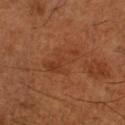Recorded during total-body skin imaging; not selected for excision or biopsy. Automated image analysis of the tile measured a nevus-likeness score of about 0/100. The recorded lesion diameter is about 5 mm. A close-up tile cropped from a whole-body skin photograph, about 15 mm across. The tile uses cross-polarized illumination. A male subject, aged around 65. The lesion is on the left lower leg.